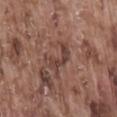Impression:
Part of a total-body skin-imaging series; this lesion was reviewed on a skin check and was not flagged for biopsy.
Context:
About 4 mm across. A region of skin cropped from a whole-body photographic capture, roughly 15 mm wide. Automated tile analysis of the lesion measured a lesion area of about 6 mm², an outline eccentricity of about 0.85 (0 = round, 1 = elongated), and a shape-asymmetry score of about 0.55 (0 = symmetric). The software also gave a lesion color around L≈41 a*≈20 b*≈23 in CIELAB and a normalized border contrast of about 7. The analysis additionally found a border-irregularity rating of about 7/10 and a color-variation rating of about 2.5/10. On the lower back. A male subject, in their mid-70s.